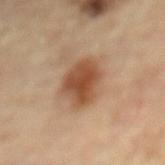  automated_metrics:
    shape_asymmetry: 0.2
    cielab_L: 48
    cielab_a: 21
    cielab_b: 32
    vs_skin_darker_L: 13.0
    vs_skin_contrast_norm: 9.5
    color_variation_0_10: 4.0
    peripheral_color_asymmetry: 1.0
    lesion_detection_confidence_0_100: 100
  site: mid back
  patient:
    sex: male
    age_approx: 85+
  image:
    source: total-body photography crop
    field_of_view_mm: 15
  lesion_size:
    long_diameter_mm_approx: 5.0
  lighting: cross-polarized